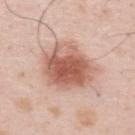Approximately 6.5 mm at its widest.
On the upper back.
Captured under white-light illumination.
A 15 mm close-up extracted from a 3D total-body photography capture.
A male subject, roughly 35 years of age.